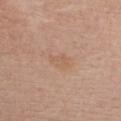Captured during whole-body skin photography for melanoma surveillance; the lesion was not biopsied. About 2.5 mm across. The subject is a female aged approximately 60. The tile uses white-light illumination. The lesion is located on the chest. A 15 mm close-up tile from a total-body photography series done for melanoma screening.